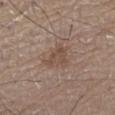Captured during whole-body skin photography for melanoma surveillance; the lesion was not biopsied.
About 3.5 mm across.
The lesion is on the front of the torso.
The tile uses white-light illumination.
This image is a 15 mm lesion crop taken from a total-body photograph.
A male patient, about 80 years old.